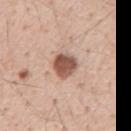Captured during whole-body skin photography for melanoma surveillance; the lesion was not biopsied. Located on the abdomen. The tile uses white-light illumination. Measured at roughly 3.5 mm in maximum diameter. A close-up tile cropped from a whole-body skin photograph, about 15 mm across. The patient is a male roughly 55 years of age.